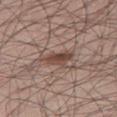notes = catalogued during a skin exam; not biopsied | body site = the right thigh | size = ≈4.5 mm | subject = male, about 55 years old | acquisition = total-body-photography crop, ~15 mm field of view | illumination = white-light illumination | image-analysis metrics = a within-lesion color-variation index near 4/10 and a peripheral color-asymmetry measure near 1.5; a classifier nevus-likeness of about 45/100.